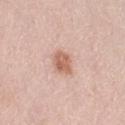follow-up: imaged on a skin check; not biopsied
patient: female, aged around 65
size: ~2.5 mm (longest diameter)
anatomic site: the left thigh
lighting: white-light
imaging modality: 15 mm crop, total-body photography
image-analysis metrics: an area of roughly 5.5 mm² and a shape-asymmetry score of about 0.15 (0 = symmetric); a detector confidence of about 100 out of 100 that the crop contains a lesion